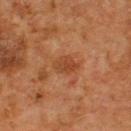Clinical summary: A region of skin cropped from a whole-body photographic capture, roughly 15 mm wide. A male patient, roughly 65 years of age. The tile uses cross-polarized illumination. The recorded lesion diameter is about 3 mm. Located on the upper back.